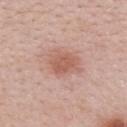{
  "biopsy_status": "not biopsied; imaged during a skin examination",
  "lesion_size": {
    "long_diameter_mm_approx": 3.5
  },
  "site": "mid back",
  "image": {
    "source": "total-body photography crop",
    "field_of_view_mm": 15
  },
  "lighting": "white-light",
  "patient": {
    "sex": "female",
    "age_approx": 30
  },
  "automated_metrics": {
    "area_mm2_approx": 7.0,
    "eccentricity": 0.7,
    "shape_asymmetry": 0.15
  }
}